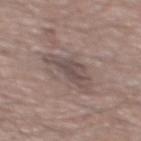The lesion was tiled from a total-body skin photograph and was not biopsied.
On the mid back.
The lesion's longest dimension is about 5.5 mm.
A region of skin cropped from a whole-body photographic capture, roughly 15 mm wide.
Captured under white-light illumination.
The patient is a male aged 53–57.
The total-body-photography lesion software estimated a lesion color around L≈48 a*≈14 b*≈18 in CIELAB, roughly 9 lightness units darker than nearby skin, and a normalized border contrast of about 7.